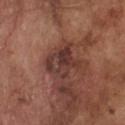Impression:
Recorded during total-body skin imaging; not selected for excision or biopsy.
Context:
This is a white-light tile. A 15 mm close-up extracted from a 3D total-body photography capture. Located on the chest. A male patient, aged approximately 75. Longest diameter approximately 4 mm. The total-body-photography lesion software estimated a border-irregularity index near 4.5/10, internal color variation of about 6 on a 0–10 scale, and radial color variation of about 2.